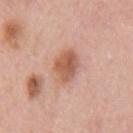Imaged during a routine full-body skin examination; the lesion was not biopsied and no histopathology is available.